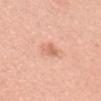automated metrics = a footprint of about 3.5 mm², a shape eccentricity near 0.75, and two-axis asymmetry of about 0.3; about 9 CIELAB-L* units darker than the surrounding skin; a border-irregularity index near 3/10, a color-variation rating of about 2.5/10, and radial color variation of about 1; a classifier nevus-likeness of about 30/100 and a detector confidence of about 100 out of 100 that the crop contains a lesion
imaging modality = total-body-photography crop, ~15 mm field of view
location = the head or neck
size = about 2.5 mm
subject = female, in their mid- to late 40s